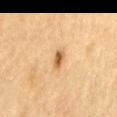{
  "biopsy_status": "not biopsied; imaged during a skin examination",
  "automated_metrics": {
    "cielab_L": 58,
    "cielab_a": 20,
    "cielab_b": 40,
    "vs_skin_darker_L": 14.0,
    "border_irregularity_0_10": 1.5,
    "color_variation_0_10": 3.5,
    "peripheral_color_asymmetry": 1.0
  },
  "patient": {
    "sex": "male",
    "age_approx": 70
  },
  "lighting": "cross-polarized",
  "image": {
    "source": "total-body photography crop",
    "field_of_view_mm": 15
  },
  "lesion_size": {
    "long_diameter_mm_approx": 2.5
  },
  "site": "mid back"
}